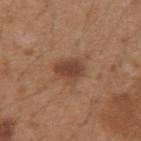On the left forearm. A female subject, roughly 30 years of age. A 15 mm close-up extracted from a 3D total-body photography capture. The lesion's longest dimension is about 3.5 mm.